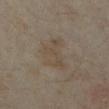Image and clinical context:
Longest diameter approximately 5 mm. This is a cross-polarized tile. An algorithmic analysis of the crop reported a shape eccentricity near 0.8 and a symmetry-axis asymmetry near 0.45. And it measured an average lesion color of about L≈43 a*≈9 b*≈23 (CIELAB) and roughly 5 lightness units darker than nearby skin. A 15 mm close-up tile from a total-body photography series done for melanoma screening. On the left lower leg. A female patient, approximately 35 years of age.